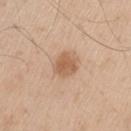{"biopsy_status": "not biopsied; imaged during a skin examination", "image": {"source": "total-body photography crop", "field_of_view_mm": 15}, "patient": {"sex": "male", "age_approx": 60}, "lighting": "white-light", "site": "left thigh", "automated_metrics": {"border_irregularity_0_10": 1.5, "color_variation_0_10": 2.5, "peripheral_color_asymmetry": 1.0, "nevus_likeness_0_100": 65}, "lesion_size": {"long_diameter_mm_approx": 3.0}}